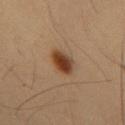workup: no biopsy performed (imaged during a skin exam) | location: the mid back | subject: male, aged 53–57 | image: total-body-photography crop, ~15 mm field of view.